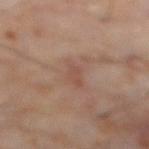biopsy_status: not biopsied; imaged during a skin examination
lesion_size:
  long_diameter_mm_approx: 3.5
site: abdomen
image:
  source: total-body photography crop
  field_of_view_mm: 15
lighting: cross-polarized
patient:
  sex: male
  age_approx: 60